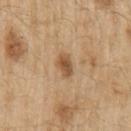notes = catalogued during a skin exam; not biopsied | tile lighting = white-light illumination | image = 15 mm crop, total-body photography | automated lesion analysis = border irregularity of about 2 on a 0–10 scale and a color-variation rating of about 3/10; a nevus-likeness score of about 80/100 and lesion-presence confidence of about 100/100 | site = the arm | patient = male, aged 68–72.